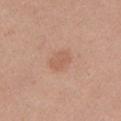subject = female, aged 33–37 | location = the left upper arm | imaging modality = ~15 mm tile from a whole-body skin photo | lighting = white-light | lesion size = about 2.5 mm.